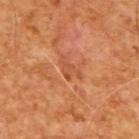follow-up: catalogued during a skin exam; not biopsied | image source: ~15 mm tile from a whole-body skin photo | TBP lesion metrics: a within-lesion color-variation index near 0.5/10 and peripheral color asymmetry of about 0 | subject: male, approximately 65 years of age | tile lighting: cross-polarized.